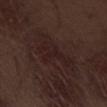biopsy status = imaged on a skin check; not biopsied
imaging modality = total-body-photography crop, ~15 mm field of view
location = the left thigh
subject = male, aged approximately 70
tile lighting = white-light illumination
diameter = about 5 mm